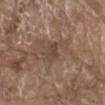Q: Was a biopsy performed?
A: catalogued during a skin exam; not biopsied
Q: Lesion size?
A: about 3 mm
Q: Where on the body is the lesion?
A: the front of the torso
Q: How was this image acquired?
A: ~15 mm tile from a whole-body skin photo
Q: Patient demographics?
A: male, aged around 80